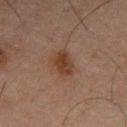This lesion was catalogued during total-body skin photography and was not selected for biopsy.
Captured under cross-polarized illumination.
The lesion's longest dimension is about 3.5 mm.
From the leg.
A male patient aged 63–67.
Cropped from a total-body skin-imaging series; the visible field is about 15 mm.